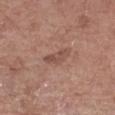notes = catalogued during a skin exam; not biopsied
patient = male, aged around 60
illumination = white-light
size = about 4 mm
image source = 15 mm crop, total-body photography
anatomic site = the right upper arm